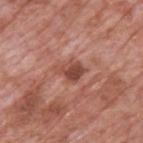Recorded during total-body skin imaging; not selected for excision or biopsy. The lesion is located on the upper back. A region of skin cropped from a whole-body photographic capture, roughly 15 mm wide. About 3.5 mm across. The tile uses white-light illumination. A male patient aged 68 to 72.